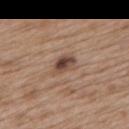<tbp_lesion>
  <biopsy_status>not biopsied; imaged during a skin examination</biopsy_status>
  <site>upper back</site>
  <image>
    <source>total-body photography crop</source>
    <field_of_view_mm>15</field_of_view_mm>
  </image>
  <lighting>white-light</lighting>
  <lesion_size>
    <long_diameter_mm_approx>3.0</long_diameter_mm_approx>
  </lesion_size>
  <patient>
    <sex>female</sex>
    <age_approx>40</age_approx>
  </patient>
</tbp_lesion>